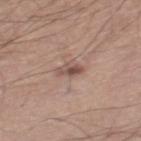| field | value |
|---|---|
| follow-up | no biopsy performed (imaged during a skin exam) |
| patient | male, aged around 40 |
| anatomic site | the right lower leg |
| image source | ~15 mm tile from a whole-body skin photo |